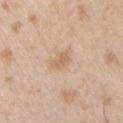  automated_metrics:
    eccentricity: 0.75
    color_variation_0_10: 1.5
  image:
    source: total-body photography crop
    field_of_view_mm: 15
  patient:
    sex: male
    age_approx: 25
  lesion_size:
    long_diameter_mm_approx: 3.0
  lighting: white-light
  site: chest